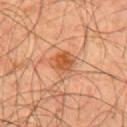Imaged during a routine full-body skin examination; the lesion was not biopsied and no histopathology is available. Cropped from a whole-body photographic skin survey; the tile spans about 15 mm. Located on the back. A male subject about 45 years old.